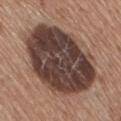The tile uses white-light illumination. Approximately 10 mm at its widest. Located on the right thigh. The patient is a female roughly 75 years of age. Cropped from a total-body skin-imaging series; the visible field is about 15 mm.